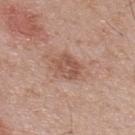Q: Where on the body is the lesion?
A: the upper back
Q: What is the imaging modality?
A: 15 mm crop, total-body photography
Q: How was the tile lit?
A: white-light illumination
Q: What did automated image analysis measure?
A: a lesion-to-skin contrast of about 6.5 (normalized; higher = more distinct); a nevus-likeness score of about 0/100
Q: Who is the patient?
A: male, aged 68–72